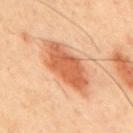follow-up = total-body-photography surveillance lesion; no biopsy | lesion diameter = about 8 mm | body site = the upper back | tile lighting = cross-polarized | TBP lesion metrics = a nevus-likeness score of about 100/100 and a lesion-detection confidence of about 100/100 | image = ~15 mm tile from a whole-body skin photo | patient = male, roughly 45 years of age.